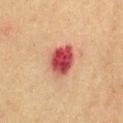biopsy status = catalogued during a skin exam; not biopsied | imaging modality = 15 mm crop, total-body photography | subject = male, aged 53–57 | location = the chest.